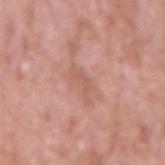image:
  source: total-body photography crop
  field_of_view_mm: 15
lighting: white-light
site: right upper arm
patient:
  sex: male
  age_approx: 60